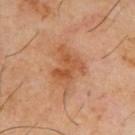The lesion was tiled from a total-body skin photograph and was not biopsied.
Cropped from a whole-body photographic skin survey; the tile spans about 15 mm.
This is a cross-polarized tile.
The total-body-photography lesion software estimated a footprint of about 13 mm², an eccentricity of roughly 0.7, and a symmetry-axis asymmetry near 0.4. The analysis additionally found a border-irregularity index near 5.5/10, internal color variation of about 4 on a 0–10 scale, and peripheral color asymmetry of about 1. The software also gave an automated nevus-likeness rating near 5 out of 100 and a detector confidence of about 100 out of 100 that the crop contains a lesion.
A male patient, aged around 65.
The recorded lesion diameter is about 6 mm.
On the back.